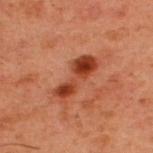Assessment:
Imaged during a routine full-body skin examination; the lesion was not biopsied and no histopathology is available.
Context:
A 15 mm close-up extracted from a 3D total-body photography capture. On the back. The subject is a male aged approximately 60.